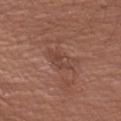The lesion was tiled from a total-body skin photograph and was not biopsied.
The patient is a male about 55 years old.
Cropped from a whole-body photographic skin survey; the tile spans about 15 mm.
This is a white-light tile.
Measured at roughly 3.5 mm in maximum diameter.
On the left upper arm.